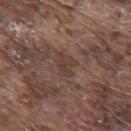Impression:
Captured during whole-body skin photography for melanoma surveillance; the lesion was not biopsied.
Image and clinical context:
The lesion is on the back. A region of skin cropped from a whole-body photographic capture, roughly 15 mm wide. The lesion's longest dimension is about 3 mm. This is a white-light tile. A male subject, aged around 75.